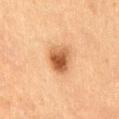| key | value |
|---|---|
| workup | catalogued during a skin exam; not biopsied |
| imaging modality | 15 mm crop, total-body photography |
| patient | female, aged 58–62 |
| diameter | about 3.5 mm |
| body site | the abdomen |
| automated metrics | a lesion area of about 8 mm² and an outline eccentricity of about 0.55 (0 = round, 1 = elongated); a nevus-likeness score of about 100/100 |
| lighting | cross-polarized illumination |